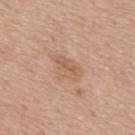The lesion was photographed on a routine skin check and not biopsied; there is no pathology result.
Located on the upper back.
About 4 mm across.
Cropped from a total-body skin-imaging series; the visible field is about 15 mm.
The tile uses white-light illumination.
The subject is a male aged around 75.